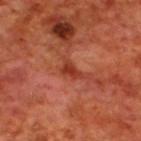Clinical impression: The lesion was photographed on a routine skin check and not biopsied; there is no pathology result. Image and clinical context: A 15 mm close-up tile from a total-body photography series done for melanoma screening. The patient is a male aged 68 to 72. The lesion is on the upper back.